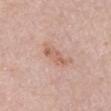Q: Was a biopsy performed?
A: total-body-photography surveillance lesion; no biopsy
Q: What is the lesion's diameter?
A: ≈3.5 mm
Q: Where on the body is the lesion?
A: the abdomen
Q: Patient demographics?
A: male, in their 80s
Q: What kind of image is this?
A: ~15 mm tile from a whole-body skin photo